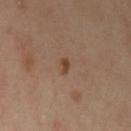Captured during whole-body skin photography for melanoma surveillance; the lesion was not biopsied. The subject is a male approximately 55 years of age. Cropped from a whole-body photographic skin survey; the tile spans about 15 mm. Automated image analysis of the tile measured an average lesion color of about L≈44 a*≈19 b*≈31 (CIELAB), a lesion–skin lightness drop of about 8, and a normalized lesion–skin contrast near 8. The analysis additionally found radial color variation of about 0.5. From the left upper arm. About 2 mm across. This is a cross-polarized tile.